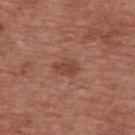– workup — catalogued during a skin exam; not biopsied
– patient — male, aged 73 to 77
– image-analysis metrics — a border-irregularity index near 2.5/10, a color-variation rating of about 1/10, and radial color variation of about 0.5; an automated nevus-likeness rating near 60 out of 100 and lesion-presence confidence of about 100/100
– image source — ~15 mm tile from a whole-body skin photo
– lesion size — ~3.5 mm (longest diameter)
– body site — the upper back